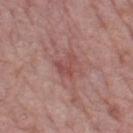The lesion was photographed on a routine skin check and not biopsied; there is no pathology result.
On the left thigh.
This is a white-light tile.
The subject is a female approximately 65 years of age.
Cropped from a total-body skin-imaging series; the visible field is about 15 mm.
Automated image analysis of the tile measured a lesion color around L≈49 a*≈26 b*≈22 in CIELAB and a normalized lesion–skin contrast near 5.5. The analysis additionally found a border-irregularity rating of about 6.5/10, a color-variation rating of about 1/10, and radial color variation of about 0. It also reported a classifier nevus-likeness of about 5/100 and lesion-presence confidence of about 100/100.